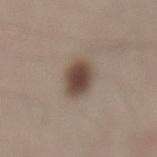The lesion was tiled from a total-body skin photograph and was not biopsied.
A roughly 15 mm field-of-view crop from a total-body skin photograph.
The patient is a male in their 30s.
From the back.